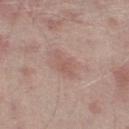| key | value |
|---|---|
| notes | imaged on a skin check; not biopsied |
| tile lighting | white-light illumination |
| site | the leg |
| TBP lesion metrics | a lesion area of about 4.5 mm² and a shape eccentricity near 0.9; a border-irregularity index near 3.5/10, internal color variation of about 1.5 on a 0–10 scale, and peripheral color asymmetry of about 0.5 |
| patient | male, in their mid- to late 60s |
| image source | total-body-photography crop, ~15 mm field of view |
| diameter | ≈3.5 mm |